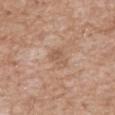Q: Was a biopsy performed?
A: total-body-photography surveillance lesion; no biopsy
Q: Automated lesion metrics?
A: a shape eccentricity near 0.8 and a symmetry-axis asymmetry near 0.3; lesion-presence confidence of about 100/100
Q: Patient demographics?
A: male, aged approximately 60
Q: What is the anatomic site?
A: the chest
Q: What is the lesion's diameter?
A: ≈3 mm
Q: What is the imaging modality?
A: ~15 mm tile from a whole-body skin photo